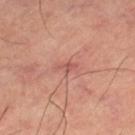biopsy_status: not biopsied; imaged during a skin examination
image:
  source: total-body photography crop
  field_of_view_mm: 15
site: left thigh
lesion_size:
  long_diameter_mm_approx: 2.5
patient:
  sex: male
  age_approx: 65
lighting: cross-polarized